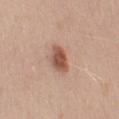notes: no biopsy performed (imaged during a skin exam)
body site: the left upper arm
tile lighting: white-light
imaging modality: ~15 mm crop, total-body skin-cancer survey
subject: male, in their 50s
automated lesion analysis: a footprint of about 6 mm², an eccentricity of roughly 0.7, and two-axis asymmetry of about 0.2; an average lesion color of about L≈53 a*≈22 b*≈29 (CIELAB) and a normalized lesion–skin contrast near 9; border irregularity of about 2 on a 0–10 scale, internal color variation of about 3.5 on a 0–10 scale, and peripheral color asymmetry of about 1